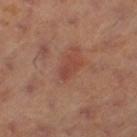Recorded during total-body skin imaging; not selected for excision or biopsy. About 2.5 mm across. The subject is a female aged 38 to 42. This is a cross-polarized tile. A 15 mm close-up extracted from a 3D total-body photography capture. On the left thigh.